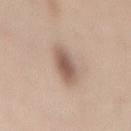No biopsy was performed on this lesion — it was imaged during a full skin examination and was not determined to be concerning. The patient is a female approximately 50 years of age. A roughly 15 mm field-of-view crop from a total-body skin photograph. Longest diameter approximately 3.5 mm. The lesion is located on the mid back. The lesion-visualizer software estimated a lesion area of about 8.5 mm² and a shape-asymmetry score of about 0.15 (0 = symmetric). And it measured a lesion–skin lightness drop of about 12. The analysis additionally found a border-irregularity rating of about 1.5/10, internal color variation of about 4 on a 0–10 scale, and radial color variation of about 1.